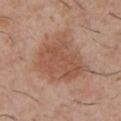Clinical impression:
Part of a total-body skin-imaging series; this lesion was reviewed on a skin check and was not flagged for biopsy.
Acquisition and patient details:
The lesion is located on the chest. An algorithmic analysis of the crop reported an area of roughly 24 mm². The analysis additionally found a border-irregularity index near 3.5/10, internal color variation of about 3.5 on a 0–10 scale, and radial color variation of about 1. A lesion tile, about 15 mm wide, cut from a 3D total-body photograph. The recorded lesion diameter is about 6 mm. The patient is a male approximately 30 years of age. Captured under white-light illumination.